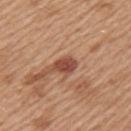<lesion>
<biopsy_status>not biopsied; imaged during a skin examination</biopsy_status>
<site>left upper arm</site>
<automated_metrics>
  <color_variation_0_10>2.5</color_variation_0_10>
  <peripheral_color_asymmetry>0.5</peripheral_color_asymmetry>
  <nevus_likeness_0_100>30</nevus_likeness_0_100>
  <lesion_detection_confidence_0_100>100</lesion_detection_confidence_0_100>
</automated_metrics>
<lighting>white-light</lighting>
<image>
  <source>total-body photography crop</source>
  <field_of_view_mm>15</field_of_view_mm>
</image>
<lesion_size>
  <long_diameter_mm_approx>2.5</long_diameter_mm_approx>
</lesion_size>
<patient>
  <sex>female</sex>
  <age_approx>40</age_approx>
</patient>
</lesion>